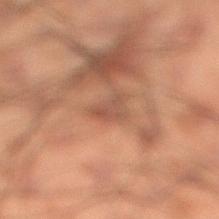| feature | finding |
|---|---|
| notes | total-body-photography surveillance lesion; no biopsy |
| size | about 3 mm |
| patient | male, roughly 50 years of age |
| image-analysis metrics | a border-irregularity rating of about 6/10 and internal color variation of about 0 on a 0–10 scale |
| location | the right lower leg |
| tile lighting | cross-polarized |
| image | 15 mm crop, total-body photography |